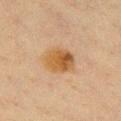Assessment: The lesion was tiled from a total-body skin photograph and was not biopsied. Image and clinical context: A female subject aged around 40. Captured under cross-polarized illumination. The lesion's longest dimension is about 4 mm. From the front of the torso. Automated image analysis of the tile measured border irregularity of about 1 on a 0–10 scale, a color-variation rating of about 7/10, and radial color variation of about 2.5. And it measured a detector confidence of about 100 out of 100 that the crop contains a lesion. A lesion tile, about 15 mm wide, cut from a 3D total-body photograph.